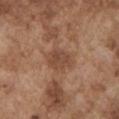Impression:
This lesion was catalogued during total-body skin photography and was not selected for biopsy.